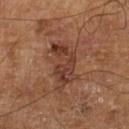Recorded during total-body skin imaging; not selected for excision or biopsy. Cropped from a total-body skin-imaging series; the visible field is about 15 mm. A male subject, in their mid-60s. The tile uses cross-polarized illumination. The total-body-photography lesion software estimated a nevus-likeness score of about 0/100 and a detector confidence of about 100 out of 100 that the crop contains a lesion.